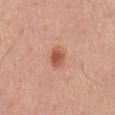Clinical summary:
From the mid back. Automated image analysis of the tile measured a shape eccentricity near 0.7 and two-axis asymmetry of about 0.3. The software also gave border irregularity of about 2.5 on a 0–10 scale, a color-variation rating of about 3.5/10, and a peripheral color-asymmetry measure near 1. And it measured a classifier nevus-likeness of about 95/100 and lesion-presence confidence of about 100/100. The tile uses white-light illumination. A 15 mm close-up extracted from a 3D total-body photography capture. The subject is a male aged 53 to 57.